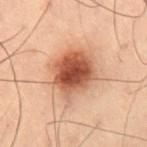Q: Was this lesion biopsied?
A: imaged on a skin check; not biopsied
Q: Who is the patient?
A: male, roughly 55 years of age
Q: What is the anatomic site?
A: the right thigh
Q: How was this image acquired?
A: ~15 mm crop, total-body skin-cancer survey